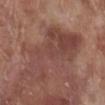Captured during whole-body skin photography for melanoma surveillance; the lesion was not biopsied. A roughly 15 mm field-of-view crop from a total-body skin photograph. From the right lower leg. A male subject, aged 68 to 72.